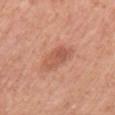Recorded during total-body skin imaging; not selected for excision or biopsy.
An algorithmic analysis of the crop reported a mean CIELAB color near L≈56 a*≈26 b*≈32, a lesion–skin lightness drop of about 9, and a normalized border contrast of about 6. And it measured a classifier nevus-likeness of about 90/100 and a lesion-detection confidence of about 100/100.
The tile uses white-light illumination.
Longest diameter approximately 3.5 mm.
The patient is a female roughly 55 years of age.
A close-up tile cropped from a whole-body skin photograph, about 15 mm across.
On the left upper arm.